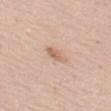Notes:
* workup: total-body-photography surveillance lesion; no biopsy
* anatomic site: the left thigh
* subject: female, aged approximately 45
* image: ~15 mm tile from a whole-body skin photo
* illumination: white-light illumination
* lesion size: about 3 mm